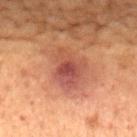Background: An algorithmic analysis of the crop reported an area of roughly 11 mm², an eccentricity of roughly 0.7, and a symmetry-axis asymmetry near 0.3. And it measured a lesion color around L≈37 a*≈22 b*≈24 in CIELAB and roughly 8 lightness units darker than nearby skin. The tile uses cross-polarized illumination. The patient is a female about 80 years old. A 15 mm crop from a total-body photograph taken for skin-cancer surveillance. The lesion is on the chest. The lesion's longest dimension is about 4.5 mm.